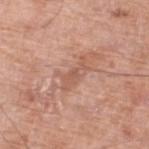Notes:
* workup: total-body-photography surveillance lesion; no biopsy
* image: ~15 mm tile from a whole-body skin photo
* automated metrics: an area of roughly 4.5 mm² and an eccentricity of roughly 0.95; a classifier nevus-likeness of about 0/100
* location: the leg
* subject: male, in their 80s